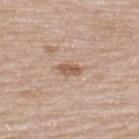Assessment:
Part of a total-body skin-imaging series; this lesion was reviewed on a skin check and was not flagged for biopsy.
Context:
A lesion tile, about 15 mm wide, cut from a 3D total-body photograph. Longest diameter approximately 2.5 mm. A male patient aged around 65. Imaged with white-light lighting. The lesion is located on the upper back.